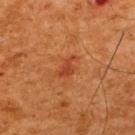{"biopsy_status": "not biopsied; imaged during a skin examination", "lighting": "cross-polarized", "image": {"source": "total-body photography crop", "field_of_view_mm": 15}, "patient": {"sex": "male", "age_approx": 65}, "site": "upper back", "lesion_size": {"long_diameter_mm_approx": 3.0}}